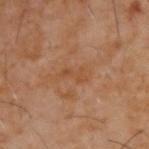<tbp_lesion>
<biopsy_status>not biopsied; imaged during a skin examination</biopsy_status>
<site>upper back</site>
<image>
  <source>total-body photography crop</source>
  <field_of_view_mm>15</field_of_view_mm>
</image>
<patient>
  <sex>male</sex>
  <age_approx>60</age_approx>
</patient>
</tbp_lesion>